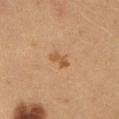{
  "biopsy_status": "not biopsied; imaged during a skin examination",
  "lesion_size": {
    "long_diameter_mm_approx": 2.5
  },
  "automated_metrics": {
    "border_irregularity_0_10": 3.0,
    "color_variation_0_10": 1.5,
    "peripheral_color_asymmetry": 0.5,
    "nevus_likeness_0_100": 10,
    "lesion_detection_confidence_0_100": 100
  },
  "image": {
    "source": "total-body photography crop",
    "field_of_view_mm": 15
  },
  "patient": {
    "sex": "female",
    "age_approx": 50
  },
  "site": "abdomen"
}